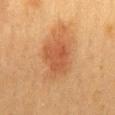location: the mid back
image source: ~15 mm crop, total-body skin-cancer survey
patient: male, in their mid- to late 30s